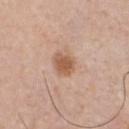{
  "biopsy_status": "not biopsied; imaged during a skin examination",
  "patient": {
    "sex": "male",
    "age_approx": 60
  },
  "lesion_size": {
    "long_diameter_mm_approx": 2.5
  },
  "automated_metrics": {
    "area_mm2_approx": 5.5,
    "eccentricity": 0.55,
    "cielab_L": 57,
    "cielab_a": 21,
    "cielab_b": 32
  },
  "lighting": "white-light",
  "site": "chest",
  "image": {
    "source": "total-body photography crop",
    "field_of_view_mm": 15
  }
}